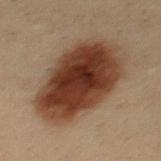Captured during whole-body skin photography for melanoma surveillance; the lesion was not biopsied.
A region of skin cropped from a whole-body photographic capture, roughly 15 mm wide.
Located on the mid back.
The subject is a male in their 30s.